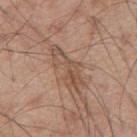Findings:
* notes · no biopsy performed (imaged during a skin exam)